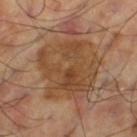notes: total-body-photography surveillance lesion; no biopsy | location: the left thigh | lesion size: ≈7 mm | lighting: cross-polarized | acquisition: ~15 mm crop, total-body skin-cancer survey | patient: male, approximately 60 years of age | automated metrics: an area of roughly 33 mm² and an outline eccentricity of about 0.35 (0 = round, 1 = elongated).